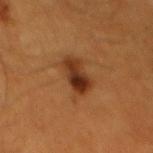{"biopsy_status": "not biopsied; imaged during a skin examination", "site": "mid back", "patient": {"sex": "male", "age_approx": 60}, "image": {"source": "total-body photography crop", "field_of_view_mm": 15}}